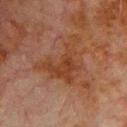Captured during whole-body skin photography for melanoma surveillance; the lesion was not biopsied.
Automated image analysis of the tile measured an area of roughly 16 mm², a shape eccentricity near 0.75, and two-axis asymmetry of about 0.65. It also reported a lesion color around L≈32 a*≈19 b*≈27 in CIELAB, about 6 CIELAB-L* units darker than the surrounding skin, and a normalized lesion–skin contrast near 6.5. It also reported a nevus-likeness score of about 0/100 and a lesion-detection confidence of about 100/100.
The patient is a male aged around 80.
On the chest.
A region of skin cropped from a whole-body photographic capture, roughly 15 mm wide.
Longest diameter approximately 6.5 mm.
This is a cross-polarized tile.